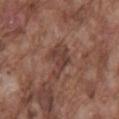Clinical impression: Captured during whole-body skin photography for melanoma surveillance; the lesion was not biopsied. Image and clinical context: The total-body-photography lesion software estimated a lesion area of about 8.5 mm², an outline eccentricity of about 0.8 (0 = round, 1 = elongated), and a shape-asymmetry score of about 0.45 (0 = symmetric). The analysis additionally found a border-irregularity rating of about 5.5/10, internal color variation of about 3 on a 0–10 scale, and radial color variation of about 1. And it measured a classifier nevus-likeness of about 0/100 and a detector confidence of about 95 out of 100 that the crop contains a lesion. A male subject, about 75 years old. Located on the mid back. The recorded lesion diameter is about 4.5 mm. A close-up tile cropped from a whole-body skin photograph, about 15 mm across. Captured under white-light illumination.